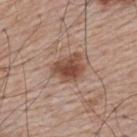Q: Lesion location?
A: the upper back
Q: How was this image acquired?
A: ~15 mm tile from a whole-body skin photo
Q: Patient demographics?
A: male, aged 63 to 67
Q: What is the lesion's diameter?
A: ~4 mm (longest diameter)
Q: Automated lesion metrics?
A: a nevus-likeness score of about 90/100 and lesion-presence confidence of about 100/100
Q: How was the tile lit?
A: white-light